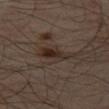Case summary:
• tile lighting — cross-polarized illumination
• body site — the left thigh
• TBP lesion metrics — an average lesion color of about L≈25 a*≈12 b*≈19 (CIELAB) and a normalized border contrast of about 8; internal color variation of about 5 on a 0–10 scale and a peripheral color-asymmetry measure near 1.5
• acquisition — total-body-photography crop, ~15 mm field of view
• subject — male, aged 63 to 67